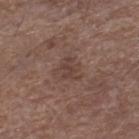A close-up tile cropped from a whole-body skin photograph, about 15 mm across. About 3 mm across. On the right lower leg. A male subject, about 70 years old.